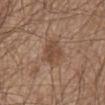The lesion was tiled from a total-body skin photograph and was not biopsied.
From the abdomen.
The recorded lesion diameter is about 3.5 mm.
Captured under white-light illumination.
The patient is a male aged approximately 65.
A region of skin cropped from a whole-body photographic capture, roughly 15 mm wide.